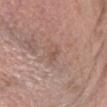notes — total-body-photography surveillance lesion; no biopsy
acquisition — ~15 mm crop, total-body skin-cancer survey
illumination — white-light
site — the head or neck
diameter — about 3 mm
patient — female, in their mid- to late 70s